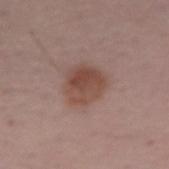Part of a total-body skin-imaging series; this lesion was reviewed on a skin check and was not flagged for biopsy. This is a white-light tile. Measured at roughly 4 mm in maximum diameter. A female patient about 55 years old. The lesion is on the arm. Automated tile analysis of the lesion measured a border-irregularity rating of about 1.5/10 and radial color variation of about 1.5. A region of skin cropped from a whole-body photographic capture, roughly 15 mm wide.